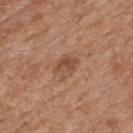Imaged during a routine full-body skin examination; the lesion was not biopsied and no histopathology is available. The tile uses white-light illumination. Longest diameter approximately 3 mm. The lesion is on the upper back. Automated tile analysis of the lesion measured an area of roughly 5 mm² and an eccentricity of roughly 0.6. The analysis additionally found a border-irregularity index near 3/10, a within-lesion color-variation index near 5/10, and radial color variation of about 2. The software also gave a classifier nevus-likeness of about 35/100 and a detector confidence of about 100 out of 100 that the crop contains a lesion. A roughly 15 mm field-of-view crop from a total-body skin photograph. A male patient in their mid- to late 60s.